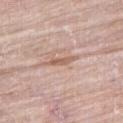This lesion was catalogued during total-body skin photography and was not selected for biopsy. The lesion-visualizer software estimated a lesion color around L≈60 a*≈20 b*≈28 in CIELAB, roughly 9 lightness units darker than nearby skin, and a normalized border contrast of about 6.5. The analysis additionally found an automated nevus-likeness rating near 0 out of 100 and lesion-presence confidence of about 80/100. The patient is a male aged 78–82. The tile uses white-light illumination. Cropped from a total-body skin-imaging series; the visible field is about 15 mm. The lesion's longest dimension is about 3.5 mm. Located on the right thigh.